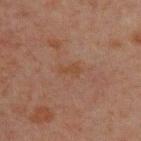Part of a total-body skin-imaging series; this lesion was reviewed on a skin check and was not flagged for biopsy. A male subject, aged around 30. This is a cross-polarized tile. Located on the upper back. The total-body-photography lesion software estimated an area of roughly 3.5 mm², a shape eccentricity near 0.85, and a symmetry-axis asymmetry near 0.35. The software also gave an average lesion color of about L≈37 a*≈17 b*≈26 (CIELAB) and a normalized lesion–skin contrast near 5.5. It also reported a nevus-likeness score of about 0/100 and lesion-presence confidence of about 100/100. Cropped from a total-body skin-imaging series; the visible field is about 15 mm. About 2.5 mm across.